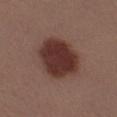Clinical impression:
Part of a total-body skin-imaging series; this lesion was reviewed on a skin check and was not flagged for biopsy.
Acquisition and patient details:
A lesion tile, about 15 mm wide, cut from a 3D total-body photograph. Longest diameter approximately 6 mm. On the right thigh. The lesion-visualizer software estimated a lesion color around L≈33 a*≈21 b*≈22 in CIELAB, roughly 14 lightness units darker than nearby skin, and a normalized border contrast of about 12. The subject is a female aged 38–42. Imaged with white-light lighting.